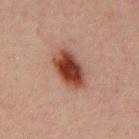Impression: The lesion was photographed on a routine skin check and not biopsied; there is no pathology result. Background: About 5 mm across. The lesion is on the mid back. The patient is a male roughly 30 years of age. The tile uses cross-polarized illumination. This image is a 15 mm lesion crop taken from a total-body photograph. Automated image analysis of the tile measured roughly 15 lightness units darker than nearby skin.